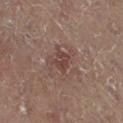No biopsy was performed on this lesion — it was imaged during a full skin examination and was not determined to be concerning. From the right leg. This is a cross-polarized tile. A female subject, approximately 80 years of age. Longest diameter approximately 2.5 mm. Automated image analysis of the tile measured an average lesion color of about L≈36 a*≈18 b*≈20 (CIELAB) and roughly 7 lightness units darker than nearby skin. The analysis additionally found border irregularity of about 3 on a 0–10 scale and a color-variation rating of about 2/10. It also reported a classifier nevus-likeness of about 0/100 and a lesion-detection confidence of about 100/100. Cropped from a whole-body photographic skin survey; the tile spans about 15 mm.